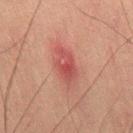{"biopsy_status": "not biopsied; imaged during a skin examination", "image": {"source": "total-body photography crop", "field_of_view_mm": 15}, "patient": {"sex": "male", "age_approx": 60}, "site": "left leg"}